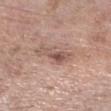Q: Was a biopsy performed?
A: imaged on a skin check; not biopsied
Q: Lesion size?
A: ~4 mm (longest diameter)
Q: What lighting was used for the tile?
A: white-light
Q: Lesion location?
A: the right forearm
Q: Patient demographics?
A: male, aged 58 to 62
Q: What kind of image is this?
A: ~15 mm tile from a whole-body skin photo